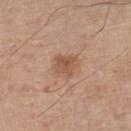This lesion was catalogued during total-body skin photography and was not selected for biopsy. The recorded lesion diameter is about 2.5 mm. The patient is a male approximately 65 years of age. An algorithmic analysis of the crop reported a footprint of about 5.5 mm² and a symmetry-axis asymmetry near 0.25. The analysis additionally found a lesion color around L≈53 a*≈20 b*≈31 in CIELAB, roughly 9 lightness units darker than nearby skin, and a normalized border contrast of about 6.5. This is a white-light tile. A lesion tile, about 15 mm wide, cut from a 3D total-body photograph. The lesion is on the leg.